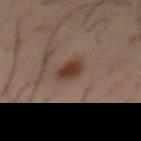Captured during whole-body skin photography for melanoma surveillance; the lesion was not biopsied.
A 15 mm close-up tile from a total-body photography series done for melanoma screening.
On the abdomen.
A male patient in their mid- to late 50s.
Imaged with cross-polarized lighting.
Approximately 3 mm at its widest.